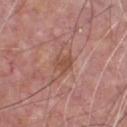Findings:
• notes: total-body-photography surveillance lesion; no biopsy